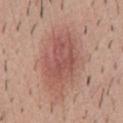Assessment: The lesion was photographed on a routine skin check and not biopsied; there is no pathology result. Acquisition and patient details: A 15 mm close-up tile from a total-body photography series done for melanoma screening. Measured at roughly 8.5 mm in maximum diameter. Captured under white-light illumination. A male subject aged 38 to 42. From the front of the torso. The lesion-visualizer software estimated an area of roughly 28 mm² and an outline eccentricity of about 0.8 (0 = round, 1 = elongated). The software also gave border irregularity of about 2.5 on a 0–10 scale, internal color variation of about 4.5 on a 0–10 scale, and a peripheral color-asymmetry measure near 1.5. It also reported a classifier nevus-likeness of about 95/100 and a detector confidence of about 100 out of 100 that the crop contains a lesion.